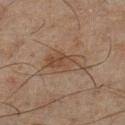Assessment:
Captured during whole-body skin photography for melanoma surveillance; the lesion was not biopsied.
Background:
A close-up tile cropped from a whole-body skin photograph, about 15 mm across. A male subject in their mid-40s. The lesion is located on the left lower leg. About 4 mm across.